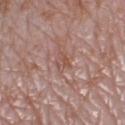Impression:
Captured during whole-body skin photography for melanoma surveillance; the lesion was not biopsied.
Image and clinical context:
A female patient, aged 58–62. On the left lower leg. A 15 mm crop from a total-body photograph taken for skin-cancer surveillance.